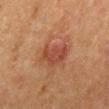A 15 mm close-up tile from a total-body photography series done for melanoma screening. A male subject approximately 50 years of age. About 4 mm across. On the mid back.